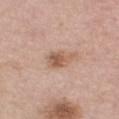This lesion was catalogued during total-body skin photography and was not selected for biopsy. A male patient, in their mid-70s. The lesion is located on the chest. A roughly 15 mm field-of-view crop from a total-body skin photograph.